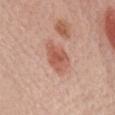Context: The lesion is located on the chest. A lesion tile, about 15 mm wide, cut from a 3D total-body photograph. This is a white-light tile. A female patient about 50 years old.